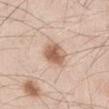{
  "biopsy_status": "not biopsied; imaged during a skin examination",
  "automated_metrics": {
    "area_mm2_approx": 8.0,
    "eccentricity": 0.35,
    "cielab_L": 61,
    "cielab_a": 19,
    "cielab_b": 30,
    "vs_skin_darker_L": 13.0,
    "vs_skin_contrast_norm": 8.5,
    "border_irregularity_0_10": 1.5,
    "lesion_detection_confidence_0_100": 100
  },
  "image": {
    "source": "total-body photography crop",
    "field_of_view_mm": 15
  },
  "patient": {
    "sex": "male",
    "age_approx": 45
  },
  "lesion_size": {
    "long_diameter_mm_approx": 3.0
  },
  "site": "right thigh",
  "lighting": "white-light"
}